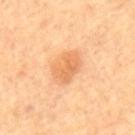About 4 mm across. On the back. A 15 mm crop from a total-body photograph taken for skin-cancer surveillance. The patient is aged 63 to 67. Captured under cross-polarized illumination.